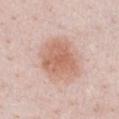Findings:
• follow-up — catalogued during a skin exam; not biopsied
• lesion size — ≈5 mm
• lighting — white-light illumination
• patient — male, in their mid- to late 20s
• body site — the chest
• acquisition — 15 mm crop, total-body photography